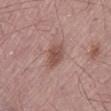{"biopsy_status": "not biopsied; imaged during a skin examination", "patient": {"sex": "male", "age_approx": 65}, "image": {"source": "total-body photography crop", "field_of_view_mm": 15}, "site": "left thigh"}